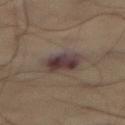Case summary:
– follow-up — total-body-photography surveillance lesion; no biopsy
– subject — male, aged around 55
– site — the abdomen
– acquisition — ~15 mm tile from a whole-body skin photo
– tile lighting — cross-polarized illumination
– lesion diameter — ~4 mm (longest diameter)